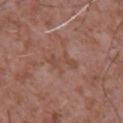Findings:
* workup — catalogued during a skin exam; not biopsied
* imaging modality — total-body-photography crop, ~15 mm field of view
* subject — male, roughly 45 years of age
* lighting — white-light
* image-analysis metrics — border irregularity of about 7.5 on a 0–10 scale and a color-variation rating of about 0/10
* location — the chest